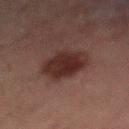<case>
<biopsy_status>not biopsied; imaged during a skin examination</biopsy_status>
<site>mid back</site>
<patient>
  <sex>male</sex>
  <age_approx>50</age_approx>
</patient>
<lighting>cross-polarized</lighting>
<image>
  <source>total-body photography crop</source>
  <field_of_view_mm>15</field_of_view_mm>
</image>
</case>